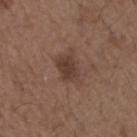Captured during whole-body skin photography for melanoma surveillance; the lesion was not biopsied.